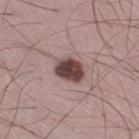The lesion was photographed on a routine skin check and not biopsied; there is no pathology result.
The lesion is located on the right thigh.
The lesion's longest dimension is about 3.5 mm.
The lesion-visualizer software estimated a footprint of about 7.5 mm², a shape eccentricity near 0.7, and a shape-asymmetry score of about 0.15 (0 = symmetric). And it measured an average lesion color of about L≈43 a*≈18 b*≈19 (CIELAB), about 19 CIELAB-L* units darker than the surrounding skin, and a normalized lesion–skin contrast near 13.5. The software also gave a border-irregularity rating of about 1.5/10 and a color-variation rating of about 3.5/10.
A region of skin cropped from a whole-body photographic capture, roughly 15 mm wide.
The subject is a male aged approximately 35.